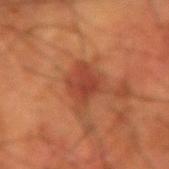Captured during whole-body skin photography for melanoma surveillance; the lesion was not biopsied. This is a cross-polarized tile. The patient is a male in their mid- to late 50s. The lesion is on the right forearm. A region of skin cropped from a whole-body photographic capture, roughly 15 mm wide.